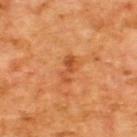workup: imaged on a skin check; not biopsied
tile lighting: cross-polarized
image: total-body-photography crop, ~15 mm field of view
lesion size: ~3.5 mm (longest diameter)
image-analysis metrics: a lesion color around L≈51 a*≈30 b*≈43 in CIELAB and about 9 CIELAB-L* units darker than the surrounding skin; a classifier nevus-likeness of about 0/100
anatomic site: the upper back
patient: male, in their mid-60s